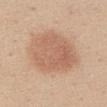Part of a total-body skin-imaging series; this lesion was reviewed on a skin check and was not flagged for biopsy.
The lesion's longest dimension is about 7 mm.
A roughly 15 mm field-of-view crop from a total-body skin photograph.
A female patient aged 43–47.
The lesion is on the abdomen.
Captured under white-light illumination.